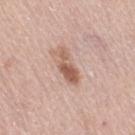{"automated_metrics": {"eccentricity": 0.9, "cielab_L": 58, "cielab_a": 20, "cielab_b": 28, "vs_skin_darker_L": 12.0, "vs_skin_contrast_norm": 8.5, "border_irregularity_0_10": 4.5, "peripheral_color_asymmetry": 0.5, "nevus_likeness_0_100": 90, "lesion_detection_confidence_0_100": 100}, "lesion_size": {"long_diameter_mm_approx": 4.5}, "lighting": "white-light", "patient": {"sex": "female", "age_approx": 65}, "site": "right thigh", "image": {"source": "total-body photography crop", "field_of_view_mm": 15}}